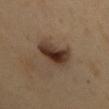A male subject in their 50s.
Approximately 4 mm at its widest.
This is a cross-polarized tile.
Located on the chest.
This image is a 15 mm lesion crop taken from a total-body photograph.